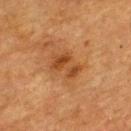No biopsy was performed on this lesion — it was imaged during a full skin examination and was not determined to be concerning.
Captured under cross-polarized illumination.
The total-body-photography lesion software estimated an area of roughly 7.5 mm², an eccentricity of roughly 0.8, and a shape-asymmetry score of about 0.4 (0 = symmetric). The software also gave a mean CIELAB color near L≈38 a*≈22 b*≈35, roughly 8 lightness units darker than nearby skin, and a normalized lesion–skin contrast near 7. It also reported a border-irregularity rating of about 4.5/10, a within-lesion color-variation index near 4/10, and radial color variation of about 1.5.
The patient is a female aged 53–57.
A roughly 15 mm field-of-view crop from a total-body skin photograph.
Approximately 4 mm at its widest.
The lesion is on the upper back.